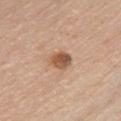* workup · no biopsy performed (imaged during a skin exam)
* patient · male, approximately 60 years of age
* location · the chest
* automated metrics · an area of roughly 5 mm², a shape eccentricity near 0.65, and a symmetry-axis asymmetry near 0.2; a lesion color around L≈54 a*≈21 b*≈32 in CIELAB, a lesion–skin lightness drop of about 14, and a normalized border contrast of about 9; a border-irregularity rating of about 2/10, a within-lesion color-variation index near 4.5/10, and peripheral color asymmetry of about 1.5
* tile lighting · white-light illumination
* lesion size · about 3 mm
* image source · 15 mm crop, total-body photography